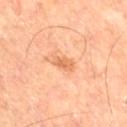{
  "biopsy_status": "not biopsied; imaged during a skin examination",
  "lighting": "cross-polarized",
  "lesion_size": {
    "long_diameter_mm_approx": 3.0
  },
  "site": "leg",
  "patient": {
    "sex": "male",
    "age_approx": 65
  },
  "image": {
    "source": "total-body photography crop",
    "field_of_view_mm": 15
  }
}